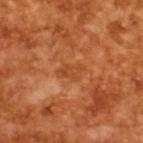Recorded during total-body skin imaging; not selected for excision or biopsy. The subject is a male aged around 65. A close-up tile cropped from a whole-body skin photograph, about 15 mm across. The tile uses cross-polarized illumination. An algorithmic analysis of the crop reported an eccentricity of roughly 0.85 and a symmetry-axis asymmetry near 0.3. The analysis additionally found a lesion–skin lightness drop of about 6 and a normalized border contrast of about 5. The software also gave lesion-presence confidence of about 100/100. The lesion's longest dimension is about 3.5 mm.